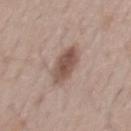Q: Was a biopsy performed?
A: total-body-photography surveillance lesion; no biopsy
Q: Who is the patient?
A: male, in their mid- to late 40s
Q: How was this image acquired?
A: ~15 mm tile from a whole-body skin photo
Q: Where on the body is the lesion?
A: the front of the torso